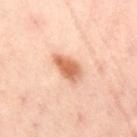The lesion was tiled from a total-body skin photograph and was not biopsied.
The lesion is located on the lower back.
A roughly 15 mm field-of-view crop from a total-body skin photograph.
Imaged with cross-polarized lighting.
A female subject, approximately 40 years of age.
The recorded lesion diameter is about 4 mm.